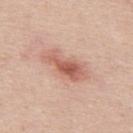workup: no biopsy performed (imaged during a skin exam) | location: the mid back | patient: male, aged around 45 | tile lighting: white-light illumination | imaging modality: ~15 mm tile from a whole-body skin photo.